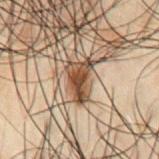Clinical impression:
Part of a total-body skin-imaging series; this lesion was reviewed on a skin check and was not flagged for biopsy.
Image and clinical context:
A male patient in their 50s. The lesion is on the chest. Automated image analysis of the tile measured a lesion color around L≈36 a*≈14 b*≈25 in CIELAB, about 14 CIELAB-L* units darker than the surrounding skin, and a normalized border contrast of about 11.5. A region of skin cropped from a whole-body photographic capture, roughly 15 mm wide. Imaged with cross-polarized lighting.